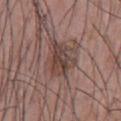Impression: Captured during whole-body skin photography for melanoma surveillance; the lesion was not biopsied.